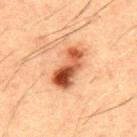<case>
  <biopsy_status>not biopsied; imaged during a skin examination</biopsy_status>
  <patient>
    <sex>male</sex>
    <age_approx>50</age_approx>
  </patient>
  <site>mid back</site>
  <image>
    <source>total-body photography crop</source>
    <field_of_view_mm>15</field_of_view_mm>
  </image>
</case>